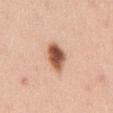{
  "biopsy_status": "not biopsied; imaged during a skin examination",
  "lesion_size": {
    "long_diameter_mm_approx": 3.5
  },
  "site": "front of the torso",
  "patient": {
    "sex": "male",
    "age_approx": 45
  },
  "image": {
    "source": "total-body photography crop",
    "field_of_view_mm": 15
  },
  "lighting": "white-light",
  "automated_metrics": {
    "cielab_L": 55,
    "cielab_a": 24,
    "cielab_b": 32,
    "vs_skin_darker_L": 20.0,
    "vs_skin_contrast_norm": 12.0,
    "border_irregularity_0_10": 2.0,
    "color_variation_0_10": 7.0,
    "peripheral_color_asymmetry": 2.5
  }
}